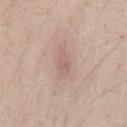Q: Was a biopsy performed?
A: no biopsy performed (imaged during a skin exam)